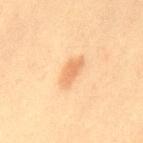No biopsy was performed on this lesion — it was imaged during a full skin examination and was not determined to be concerning.
A lesion tile, about 15 mm wide, cut from a 3D total-body photograph.
The patient is a male in their 60s.
Approximately 4 mm at its widest.
From the mid back.
An algorithmic analysis of the crop reported a nevus-likeness score of about 80/100 and lesion-presence confidence of about 100/100.